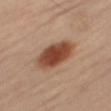Q: What kind of image is this?
A: ~15 mm crop, total-body skin-cancer survey
Q: Lesion location?
A: the right thigh
Q: Who is the patient?
A: female, approximately 65 years of age